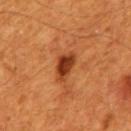Findings:
• subject · male, in their 60s
• illumination · cross-polarized
• lesion diameter · ~4 mm (longest diameter)
• location · the mid back
• image source · ~15 mm crop, total-body skin-cancer survey
• automated metrics · a shape-asymmetry score of about 0.25 (0 = symmetric); a classifier nevus-likeness of about 90/100 and lesion-presence confidence of about 100/100